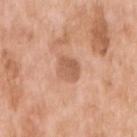Imaged during a routine full-body skin examination; the lesion was not biopsied and no histopathology is available.
A female subject, aged approximately 75.
Cropped from a total-body skin-imaging series; the visible field is about 15 mm.
The lesion is located on the right upper arm.
This is a white-light tile.
Automated tile analysis of the lesion measured an average lesion color of about L≈59 a*≈23 b*≈32 (CIELAB), about 10 CIELAB-L* units darker than the surrounding skin, and a lesion-to-skin contrast of about 6.5 (normalized; higher = more distinct). And it measured a detector confidence of about 100 out of 100 that the crop contains a lesion.
Longest diameter approximately 3 mm.